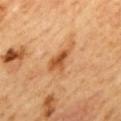A male subject approximately 60 years of age. On the mid back. A 15 mm crop from a total-body photograph taken for skin-cancer surveillance.